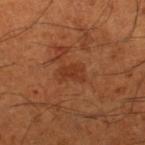Part of a total-body skin-imaging series; this lesion was reviewed on a skin check and was not flagged for biopsy. The lesion is on the right lower leg. The subject is a male in their mid-60s. The tile uses cross-polarized illumination. This image is a 15 mm lesion crop taken from a total-body photograph. Automated image analysis of the tile measured a shape eccentricity near 0.8 and two-axis asymmetry of about 0.3. The analysis additionally found a mean CIELAB color near L≈36 a*≈27 b*≈34 and a normalized border contrast of about 6. And it measured border irregularity of about 4 on a 0–10 scale and radial color variation of about 0. And it measured a nevus-likeness score of about 0/100 and a detector confidence of about 100 out of 100 that the crop contains a lesion.